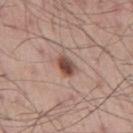This lesion was catalogued during total-body skin photography and was not selected for biopsy. Cropped from a total-body skin-imaging series; the visible field is about 15 mm. On the chest. The subject is a male aged 53–57. Imaged with white-light lighting.